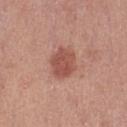The lesion was tiled from a total-body skin photograph and was not biopsied. Cropped from a whole-body photographic skin survey; the tile spans about 15 mm. The lesion is on the left thigh. The tile uses white-light illumination. A female subject about 40 years old.